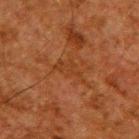Q: Was this lesion biopsied?
A: imaged on a skin check; not biopsied
Q: What kind of image is this?
A: 15 mm crop, total-body photography
Q: Where on the body is the lesion?
A: the upper back
Q: Automated lesion metrics?
A: an outline eccentricity of about 0.9 (0 = round, 1 = elongated); a border-irregularity rating of about 8/10, internal color variation of about 1 on a 0–10 scale, and peripheral color asymmetry of about 0; a nevus-likeness score of about 0/100 and a lesion-detection confidence of about 80/100
Q: Who is the patient?
A: male, roughly 60 years of age
Q: What is the lesion's diameter?
A: ~4 mm (longest diameter)
Q: Illumination type?
A: cross-polarized illumination